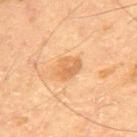Captured during whole-body skin photography for melanoma surveillance; the lesion was not biopsied. The tile uses cross-polarized illumination. The lesion's longest dimension is about 3.5 mm. The subject is a male about 60 years old. This image is a 15 mm lesion crop taken from a total-body photograph. The lesion-visualizer software estimated a lesion-detection confidence of about 100/100.